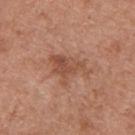workup = imaged on a skin check; not biopsied
TBP lesion metrics = a footprint of about 8.5 mm²; a lesion color around L≈50 a*≈23 b*≈31 in CIELAB
lesion diameter = about 5 mm
imaging modality = 15 mm crop, total-body photography
body site = the upper back
subject = male, about 55 years old
lighting = white-light